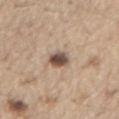Assessment: The lesion was photographed on a routine skin check and not biopsied; there is no pathology result. Image and clinical context: From the abdomen. A 15 mm close-up extracted from a 3D total-body photography capture. A male subject, in their 70s. The recorded lesion diameter is about 3 mm.